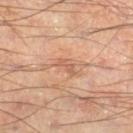<tbp_lesion>
<biopsy_status>not biopsied; imaged during a skin examination</biopsy_status>
<patient>
  <sex>male</sex>
  <age_approx>55</age_approx>
</patient>
<image>
  <source>total-body photography crop</source>
  <field_of_view_mm>15</field_of_view_mm>
</image>
<lighting>cross-polarized</lighting>
<lesion_size>
  <long_diameter_mm_approx>2.5</long_diameter_mm_approx>
</lesion_size>
<site>right lower leg</site>
</tbp_lesion>